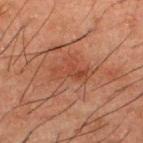  biopsy_status: not biopsied; imaged during a skin examination
  site: upper back
  image:
    source: total-body photography crop
    field_of_view_mm: 15
  patient:
    sex: male
    age_approx: 50